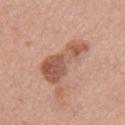{
  "biopsy_status": "not biopsied; imaged during a skin examination",
  "automated_metrics": {
    "area_mm2_approx": 15.0,
    "shape_asymmetry": 0.45,
    "cielab_L": 55,
    "cielab_a": 23,
    "cielab_b": 30,
    "vs_skin_darker_L": 12.0,
    "vs_skin_contrast_norm": 8.0,
    "border_irregularity_0_10": 5.5,
    "color_variation_0_10": 6.0,
    "peripheral_color_asymmetry": 2.0,
    "nevus_likeness_0_100": 5
  },
  "lesion_size": {
    "long_diameter_mm_approx": 6.5
  },
  "patient": {
    "sex": "female",
    "age_approx": 35
  },
  "image": {
    "source": "total-body photography crop",
    "field_of_view_mm": 15
  },
  "site": "left upper arm",
  "lighting": "white-light"
}